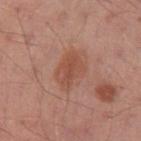Recorded during total-body skin imaging; not selected for excision or biopsy. Measured at roughly 4 mm in maximum diameter. A lesion tile, about 15 mm wide, cut from a 3D total-body photograph. The lesion-visualizer software estimated a classifier nevus-likeness of about 30/100. A male patient, aged around 30. Located on the left upper arm.